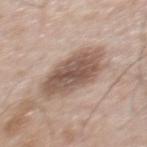<tbp_lesion>
<biopsy_status>not biopsied; imaged during a skin examination</biopsy_status>
<lighting>white-light</lighting>
<image>
  <source>total-body photography crop</source>
  <field_of_view_mm>15</field_of_view_mm>
</image>
<automated_metrics>
  <cielab_L>54</cielab_L>
  <cielab_a>16</cielab_a>
  <cielab_b>25</cielab_b>
  <vs_skin_contrast_norm>9.0</vs_skin_contrast_norm>
  <border_irregularity_0_10>2.5</border_irregularity_0_10>
  <color_variation_0_10>4.5</color_variation_0_10>
  <peripheral_color_asymmetry>1.5</peripheral_color_asymmetry>
  <nevus_likeness_0_100>5</nevus_likeness_0_100>
</automated_metrics>
<site>back</site>
<lesion_size>
  <long_diameter_mm_approx>6.5</long_diameter_mm_approx>
</lesion_size>
<patient>
  <sex>male</sex>
  <age_approx>55</age_approx>
</patient>
</tbp_lesion>